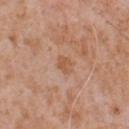Q: What kind of image is this?
A: ~15 mm tile from a whole-body skin photo
Q: How was the tile lit?
A: white-light
Q: How large is the lesion?
A: about 2.5 mm
Q: Lesion location?
A: the chest
Q: Who is the patient?
A: male, aged around 65